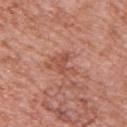| key | value |
|---|---|
| notes | no biopsy performed (imaged during a skin exam) |
| size | ~3.5 mm (longest diameter) |
| imaging modality | ~15 mm crop, total-body skin-cancer survey |
| location | the upper back |
| tile lighting | white-light illumination |
| subject | male, roughly 60 years of age |
| image-analysis metrics | a lesion area of about 4.5 mm² and two-axis asymmetry of about 0.45; a normalized lesion–skin contrast near 5.5; a border-irregularity rating of about 6/10 and internal color variation of about 2.5 on a 0–10 scale |